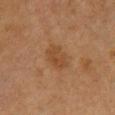{
  "biopsy_status": "not biopsied; imaged during a skin examination",
  "lighting": "cross-polarized",
  "automated_metrics": {
    "area_mm2_approx": 7.0,
    "eccentricity": 0.75,
    "cielab_L": 38,
    "cielab_a": 18,
    "cielab_b": 31,
    "vs_skin_darker_L": 6.0,
    "nevus_likeness_0_100": 10
  },
  "patient": {
    "sex": "female",
    "age_approx": 55
  },
  "image": {
    "source": "total-body photography crop",
    "field_of_view_mm": 15
  },
  "site": "chest"
}